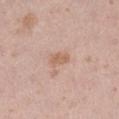The lesion was tiled from a total-body skin photograph and was not biopsied.
Automated tile analysis of the lesion measured a footprint of about 4 mm², a shape eccentricity near 0.85, and a shape-asymmetry score of about 0.25 (0 = symmetric). And it measured an automated nevus-likeness rating near 10 out of 100.
From the right lower leg.
A 15 mm crop from a total-body photograph taken for skin-cancer surveillance.
The tile uses white-light illumination.
The subject is a female roughly 30 years of age.